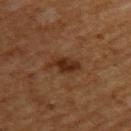The lesion was tiled from a total-body skin photograph and was not biopsied. Captured under cross-polarized illumination. The recorded lesion diameter is about 4 mm. From the front of the torso. A 15 mm close-up extracted from a 3D total-body photography capture. A male subject, approximately 60 years of age.